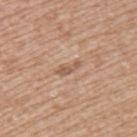Clinical impression:
Imaged during a routine full-body skin examination; the lesion was not biopsied and no histopathology is available.
Acquisition and patient details:
A region of skin cropped from a whole-body photographic capture, roughly 15 mm wide. The lesion is on the upper back. A female subject aged 38–42.